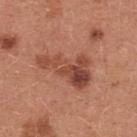Q: Lesion location?
A: the chest
Q: How was this image acquired?
A: 15 mm crop, total-body photography
Q: How large is the lesion?
A: ≈6.5 mm
Q: What are the patient's age and sex?
A: female, in their mid- to late 30s
Q: How was the tile lit?
A: white-light
Q: Automated lesion metrics?
A: an area of roughly 16 mm², an eccentricity of roughly 0.85, and two-axis asymmetry of about 0.45